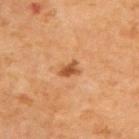No biopsy was performed on this lesion — it was imaged during a full skin examination and was not determined to be concerning. This image is a 15 mm lesion crop taken from a total-body photograph. Automated image analysis of the tile measured an average lesion color of about L≈52 a*≈25 b*≈40 (CIELAB), about 12 CIELAB-L* units darker than the surrounding skin, and a lesion-to-skin contrast of about 8 (normalized; higher = more distinct). The analysis additionally found a border-irregularity index near 3/10, a within-lesion color-variation index near 2/10, and a peripheral color-asymmetry measure near 0.5. The analysis additionally found an automated nevus-likeness rating near 60 out of 100 and a detector confidence of about 100 out of 100 that the crop contains a lesion. The tile uses cross-polarized illumination. On the upper back. A male patient aged around 65. About 2.5 mm across.